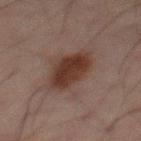biopsy_status: not biopsied; imaged during a skin examination
patient:
  sex: male
  age_approx: 65
lighting: cross-polarized
image:
  source: total-body photography crop
  field_of_view_mm: 15
site: mid back
lesion_size:
  long_diameter_mm_approx: 5.0